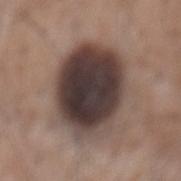Assessment: Part of a total-body skin-imaging series; this lesion was reviewed on a skin check and was not flagged for biopsy. Image and clinical context: Captured under white-light illumination. This image is a 15 mm lesion crop taken from a total-body photograph. Measured at roughly 8.5 mm in maximum diameter. On the mid back. The subject is a male aged approximately 60.